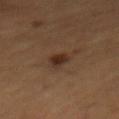Part of a total-body skin-imaging series; this lesion was reviewed on a skin check and was not flagged for biopsy. A male patient aged around 60. A roughly 15 mm field-of-view crop from a total-body skin photograph. The lesion is located on the mid back.